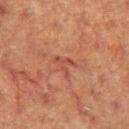notes = total-body-photography surveillance lesion; no biopsy
subject = female, in their 40s
image = total-body-photography crop, ~15 mm field of view
lighting = cross-polarized
lesion diameter = about 3 mm
body site = the leg